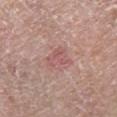biopsy_status: not biopsied; imaged during a skin examination
image:
  source: total-body photography crop
  field_of_view_mm: 15
site: left lower leg
automated_metrics:
  area_mm2_approx: 6.0
  color_variation_0_10: 3.0
  peripheral_color_asymmetry: 1.0
lesion_size:
  long_diameter_mm_approx: 3.5
lighting: white-light
patient:
  sex: male
  age_approx: 80